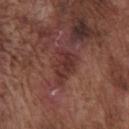Assessment: Recorded during total-body skin imaging; not selected for excision or biopsy. Image and clinical context: From the chest. A roughly 15 mm field-of-view crop from a total-body skin photograph. The total-body-photography lesion software estimated an average lesion color of about L≈34 a*≈23 b*≈22 (CIELAB), roughly 8 lightness units darker than nearby skin, and a lesion-to-skin contrast of about 7 (normalized; higher = more distinct). The software also gave border irregularity of about 3.5 on a 0–10 scale, a within-lesion color-variation index near 4/10, and radial color variation of about 1. The analysis additionally found a classifier nevus-likeness of about 5/100. The lesion's longest dimension is about 4.5 mm. A male subject, aged approximately 75.